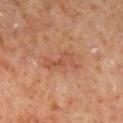Captured during whole-body skin photography for melanoma surveillance; the lesion was not biopsied. This image is a 15 mm lesion crop taken from a total-body photograph. A male subject, aged approximately 60. Automated image analysis of the tile measured a footprint of about 6 mm² and an outline eccentricity of about 0.95 (0 = round, 1 = elongated). And it measured a lesion color around L≈49 a*≈24 b*≈31 in CIELAB, about 7 CIELAB-L* units darker than the surrounding skin, and a normalized lesion–skin contrast near 5. It also reported a border-irregularity rating of about 7/10, internal color variation of about 2 on a 0–10 scale, and a peripheral color-asymmetry measure near 0.5. The analysis additionally found a detector confidence of about 100 out of 100 that the crop contains a lesion. This is a cross-polarized tile. The lesion is located on the right lower leg.